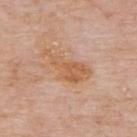– anatomic site: the upper back
– diameter: about 6 mm
– image source: ~15 mm crop, total-body skin-cancer survey
– tile lighting: white-light
– TBP lesion metrics: a lesion color around L≈60 a*≈21 b*≈35 in CIELAB, about 8 CIELAB-L* units darker than the surrounding skin, and a lesion-to-skin contrast of about 7 (normalized; higher = more distinct); border irregularity of about 6 on a 0–10 scale, a within-lesion color-variation index near 3.5/10, and radial color variation of about 1; a nevus-likeness score of about 0/100 and a detector confidence of about 100 out of 100 that the crop contains a lesion
– subject: male, in their mid- to late 70s